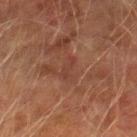Findings:
* follow-up — total-body-photography surveillance lesion; no biopsy
* acquisition — ~15 mm crop, total-body skin-cancer survey
* patient — male, aged around 75
* location — the right lower leg